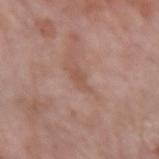No biopsy was performed on this lesion — it was imaged during a full skin examination and was not determined to be concerning. This is a white-light tile. A female patient about 60 years old. On the left forearm. A close-up tile cropped from a whole-body skin photograph, about 15 mm across. The lesion's longest dimension is about 3 mm.